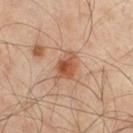Case summary:
- workup: catalogued during a skin exam; not biopsied
- subject: male, aged 58–62
- site: the right thigh
- acquisition: ~15 mm tile from a whole-body skin photo